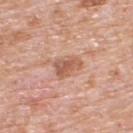{"biopsy_status": "not biopsied; imaged during a skin examination", "automated_metrics": {"cielab_L": 58, "cielab_a": 23, "cielab_b": 31, "vs_skin_darker_L": 11.0, "vs_skin_contrast_norm": 7.0, "border_irregularity_0_10": 3.0, "color_variation_0_10": 4.0, "peripheral_color_asymmetry": 1.5, "nevus_likeness_0_100": 0}, "patient": {"sex": "male", "age_approx": 80}, "image": {"source": "total-body photography crop", "field_of_view_mm": 15}, "lesion_size": {"long_diameter_mm_approx": 3.5}, "site": "upper back"}